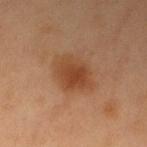Q: Was this lesion biopsied?
A: total-body-photography surveillance lesion; no biopsy
Q: Who is the patient?
A: female, aged 48 to 52
Q: Where on the body is the lesion?
A: the left thigh
Q: How was this image acquired?
A: total-body-photography crop, ~15 mm field of view
Q: What lighting was used for the tile?
A: cross-polarized illumination
Q: Automated lesion metrics?
A: a lesion area of about 13 mm², an outline eccentricity of about 0.6 (0 = round, 1 = elongated), and a shape-asymmetry score of about 0.2 (0 = symmetric); a mean CIELAB color near L≈37 a*≈19 b*≈29, roughly 8 lightness units darker than nearby skin, and a lesion-to-skin contrast of about 7 (normalized; higher = more distinct); an automated nevus-likeness rating near 95 out of 100
Q: Lesion size?
A: ~4.5 mm (longest diameter)